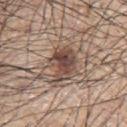lesion diameter: about 4 mm
tile lighting: white-light
automated metrics: a lesion color around L≈46 a*≈17 b*≈23 in CIELAB, roughly 13 lightness units darker than nearby skin, and a lesion-to-skin contrast of about 10 (normalized; higher = more distinct)
image: total-body-photography crop, ~15 mm field of view
anatomic site: the left upper arm
subject: male, in their 70s